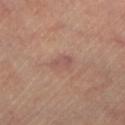{"image": {"source": "total-body photography crop", "field_of_view_mm": 15}, "automated_metrics": {"cielab_L": 53, "cielab_a": 21, "cielab_b": 23, "vs_skin_darker_L": 7.0, "vs_skin_contrast_norm": 5.5}, "site": "right leg", "patient": {"sex": "female", "age_approx": 60}, "lighting": "cross-polarized"}